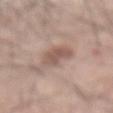Imaged during a routine full-body skin examination; the lesion was not biopsied and no histopathology is available. A male patient approximately 70 years of age. Imaged with white-light lighting. The lesion-visualizer software estimated a lesion color around L≈55 a*≈18 b*≈24 in CIELAB and a normalized lesion–skin contrast near 7. The lesion is located on the mid back. A 15 mm crop from a total-body photograph taken for skin-cancer surveillance. Longest diameter approximately 5 mm.